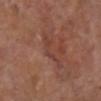The lesion was photographed on a routine skin check and not biopsied; there is no pathology result.
Imaged with cross-polarized lighting.
The lesion is located on the leg.
A region of skin cropped from a whole-body photographic capture, roughly 15 mm wide.
The lesion's longest dimension is about 5 mm.
The total-body-photography lesion software estimated a lesion area of about 5.5 mm², a shape eccentricity near 0.95, and a shape-asymmetry score of about 0.65 (0 = symmetric). It also reported an average lesion color of about L≈41 a*≈23 b*≈25 (CIELAB). The software also gave border irregularity of about 9 on a 0–10 scale and a within-lesion color-variation index near 0/10. It also reported a lesion-detection confidence of about 80/100.